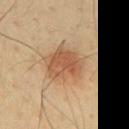biopsy_status: not biopsied; imaged during a skin examination
lesion_size:
  long_diameter_mm_approx: 4.5
site: mid back
automated_metrics:
  area_mm2_approx: 14.0
  eccentricity: 0.3
  border_irregularity_0_10: 2.5
  color_variation_0_10: 4.0
  peripheral_color_asymmetry: 1.0
  nevus_likeness_0_100: 95
  lesion_detection_confidence_0_100: 100
patient:
  sex: male
  age_approx: 45
lighting: cross-polarized
image:
  source: total-body photography crop
  field_of_view_mm: 15